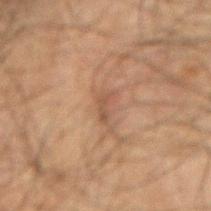Recorded during total-body skin imaging; not selected for excision or biopsy.
Measured at roughly 3 mm in maximum diameter.
Imaged with cross-polarized lighting.
Automated image analysis of the tile measured an automated nevus-likeness rating near 0 out of 100 and a lesion-detection confidence of about 55/100.
From the right upper arm.
A 15 mm close-up tile from a total-body photography series done for melanoma screening.
A male patient, approximately 65 years of age.